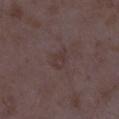TBP lesion metrics: a lesion area of about 4 mm² and an outline eccentricity of about 0.75 (0 = round, 1 = elongated); a nevus-likeness score of about 0/100 and a lesion-detection confidence of about 100/100
subject: female, aged 33 to 37
body site: the leg
tile lighting: white-light
acquisition: ~15 mm tile from a whole-body skin photo
lesion size: ~2.5 mm (longest diameter)